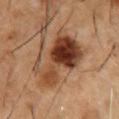The lesion was tiled from a total-body skin photograph and was not biopsied. Located on the chest. Captured under cross-polarized illumination. A region of skin cropped from a whole-body photographic capture, roughly 15 mm wide. A male subject aged 53–57. The lesion-visualizer software estimated a lesion area of about 26 mm² and an outline eccentricity of about 0.85 (0 = round, 1 = elongated). The analysis additionally found a mean CIELAB color near L≈41 a*≈22 b*≈32, roughly 15 lightness units darker than nearby skin, and a lesion-to-skin contrast of about 11.5 (normalized; higher = more distinct). The analysis additionally found a lesion-detection confidence of about 100/100. The lesion's longest dimension is about 7.5 mm.